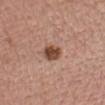The lesion is located on the front of the torso. The total-body-photography lesion software estimated an area of roughly 5 mm², an outline eccentricity of about 0.7 (0 = round, 1 = elongated), and two-axis asymmetry of about 0.15. The analysis additionally found lesion-presence confidence of about 100/100. A male subject roughly 50 years of age. The lesion's longest dimension is about 3 mm. This image is a 15 mm lesion crop taken from a total-body photograph.